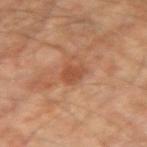Cropped from a total-body skin-imaging series; the visible field is about 15 mm.
On the arm.
This is a cross-polarized tile.
The lesion-visualizer software estimated a lesion area of about 4 mm² and a shape eccentricity near 0.65. It also reported an average lesion color of about L≈49 a*≈25 b*≈34 (CIELAB), about 9 CIELAB-L* units darker than the surrounding skin, and a normalized border contrast of about 7. The analysis additionally found internal color variation of about 1.5 on a 0–10 scale.
A male subject, aged approximately 65.
About 2.5 mm across.